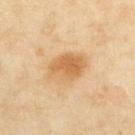Impression:
Recorded during total-body skin imaging; not selected for excision or biopsy.
Background:
Imaged with cross-polarized lighting. A roughly 15 mm field-of-view crop from a total-body skin photograph. On the upper back. A female patient, aged approximately 35.